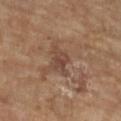Context:
On the left lower leg. A close-up tile cropped from a whole-body skin photograph, about 15 mm across. The patient is a female aged 68 to 72. Longest diameter approximately 3 mm. The tile uses cross-polarized illumination.